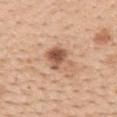biopsy status = no biopsy performed (imaged during a skin exam); size = ~4.5 mm (longest diameter); anatomic site = the mid back; subject = female, about 65 years old; image source = total-body-photography crop, ~15 mm field of view; lighting = white-light; image-analysis metrics = an area of roughly 8.5 mm², an eccentricity of roughly 0.75, and a symmetry-axis asymmetry near 0.25.